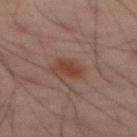| field | value |
|---|---|
| follow-up | no biopsy performed (imaged during a skin exam) |
| diameter | ≈2.5 mm |
| body site | the mid back |
| imaging modality | 15 mm crop, total-body photography |
| image-analysis metrics | an average lesion color of about L≈31 a*≈18 b*≈22 (CIELAB), about 7 CIELAB-L* units darker than the surrounding skin, and a normalized border contrast of about 8; a border-irregularity rating of about 2/10 and a within-lesion color-variation index near 1.5/10 |
| patient | male, approximately 65 years of age |